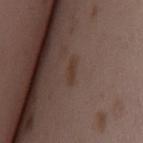Part of a total-body skin-imaging series; this lesion was reviewed on a skin check and was not flagged for biopsy. Located on the back. The tile uses white-light illumination. A female subject, roughly 35 years of age. This image is a 15 mm lesion crop taken from a total-body photograph. About 3 mm across.